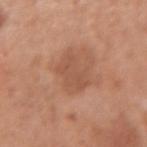Assessment:
Recorded during total-body skin imaging; not selected for excision or biopsy.
Image and clinical context:
Approximately 4 mm at its widest. The patient is a female aged approximately 50. This image is a 15 mm lesion crop taken from a total-body photograph. The total-body-photography lesion software estimated a border-irregularity index near 5/10, internal color variation of about 2 on a 0–10 scale, and a peripheral color-asymmetry measure near 0.5. And it measured an automated nevus-likeness rating near 0 out of 100 and lesion-presence confidence of about 100/100. The lesion is on the left forearm.